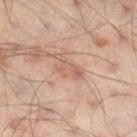Case summary:
– workup — catalogued during a skin exam; not biopsied
– illumination — cross-polarized illumination
– patient — male, aged around 65
– site — the leg
– image-analysis metrics — a lesion area of about 5 mm², an eccentricity of roughly 0.9, and a shape-asymmetry score of about 0.55 (0 = symmetric); a mean CIELAB color near L≈57 a*≈20 b*≈27, about 7 CIELAB-L* units darker than the surrounding skin, and a lesion-to-skin contrast of about 5 (normalized; higher = more distinct); a border-irregularity rating of about 6.5/10, a within-lesion color-variation index near 2.5/10, and radial color variation of about 0
– image source — ~15 mm tile from a whole-body skin photo
– diameter — about 4 mm